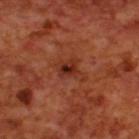Q: Was this lesion biopsied?
A: imaged on a skin check; not biopsied
Q: How was the tile lit?
A: cross-polarized
Q: What did automated image analysis measure?
A: a lesion area of about 6 mm², an eccentricity of roughly 0.7, and two-axis asymmetry of about 0.35; a border-irregularity index near 4/10 and radial color variation of about 2.5; a detector confidence of about 100 out of 100 that the crop contains a lesion
Q: Where on the body is the lesion?
A: the upper back
Q: What is the lesion's diameter?
A: ~3.5 mm (longest diameter)
Q: What is the imaging modality?
A: 15 mm crop, total-body photography
Q: Patient demographics?
A: male, about 70 years old